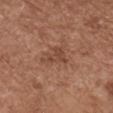Captured during whole-body skin photography for melanoma surveillance; the lesion was not biopsied.
A female subject approximately 75 years of age.
On the chest.
Cropped from a total-body skin-imaging series; the visible field is about 15 mm.